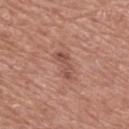notes = total-body-photography surveillance lesion; no biopsy | subject = male, roughly 75 years of age | image source = total-body-photography crop, ~15 mm field of view | anatomic site = the mid back.